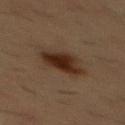Recorded during total-body skin imaging; not selected for excision or biopsy.
A 15 mm close-up tile from a total-body photography series done for melanoma screening.
The patient is a male aged 53–57.
About 4.5 mm across.
Imaged with cross-polarized lighting.
From the front of the torso.